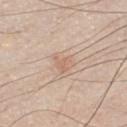Context: A male subject aged approximately 45. Located on the front of the torso. The lesion's longest dimension is about 2.5 mm. A lesion tile, about 15 mm wide, cut from a 3D total-body photograph.